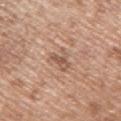Impression:
No biopsy was performed on this lesion — it was imaged during a full skin examination and was not determined to be concerning.
Clinical summary:
A 15 mm close-up tile from a total-body photography series done for melanoma screening. A male patient aged 48 to 52. Located on the right upper arm.